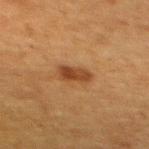biopsy status: catalogued during a skin exam; not biopsied
subject: female, approximately 60 years of age
body site: the upper back
imaging modality: 15 mm crop, total-body photography
tile lighting: cross-polarized illumination